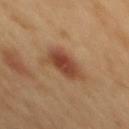Assessment:
The lesion was photographed on a routine skin check and not biopsied; there is no pathology result.
Clinical summary:
The lesion's longest dimension is about 4 mm. This image is a 15 mm lesion crop taken from a total-body photograph. A male patient roughly 50 years of age. The lesion is on the mid back.